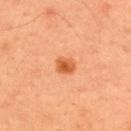* subject — male, aged 33 to 37
* automated metrics — an area of roughly 4 mm², a shape eccentricity near 0.65, and two-axis asymmetry of about 0.2; a mean CIELAB color near L≈57 a*≈31 b*≈43 and a lesion–skin lightness drop of about 12
* image — 15 mm crop, total-body photography
* diameter — ~2.5 mm (longest diameter)
* site — the back